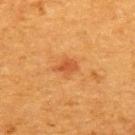No biopsy was performed on this lesion — it was imaged during a full skin examination and was not determined to be concerning.
About 3 mm across.
A 15 mm close-up extracted from a 3D total-body photography capture.
From the upper back.
A female subject, aged approximately 40.
The tile uses cross-polarized illumination.